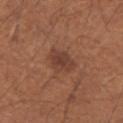lesion size: ~3 mm (longest diameter) | location: the arm | automated metrics: a mean CIELAB color near L≈40 a*≈22 b*≈27 and roughly 8 lightness units darker than nearby skin; internal color variation of about 2.5 on a 0–10 scale and radial color variation of about 1; a nevus-likeness score of about 65/100 and a detector confidence of about 100 out of 100 that the crop contains a lesion | lighting: white-light | patient: male, aged approximately 55 | imaging modality: 15 mm crop, total-body photography.